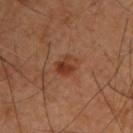Imaged during a routine full-body skin examination; the lesion was not biopsied and no histopathology is available. On the upper back. A male patient, aged 58–62. This image is a 15 mm lesion crop taken from a total-body photograph.